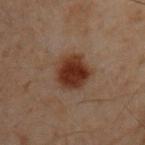Assessment: The lesion was tiled from a total-body skin photograph and was not biopsied. Acquisition and patient details: The lesion-visualizer software estimated an area of roughly 12 mm², an outline eccentricity of about 0.5 (0 = round, 1 = elongated), and a symmetry-axis asymmetry near 0.15. The software also gave a lesion color around L≈27 a*≈18 b*≈24 in CIELAB and a normalized lesion–skin contrast near 11.5. The analysis additionally found a within-lesion color-variation index near 3.5/10. And it measured a lesion-detection confidence of about 100/100. Measured at roughly 4.5 mm in maximum diameter. A male patient approximately 50 years of age. This is a cross-polarized tile. The lesion is on the upper back. A lesion tile, about 15 mm wide, cut from a 3D total-body photograph.